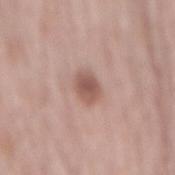notes: imaged on a skin check; not biopsied | body site: the mid back | lighting: white-light | TBP lesion metrics: a normalized border contrast of about 8; border irregularity of about 2 on a 0–10 scale and a color-variation rating of about 2.5/10 | image: ~15 mm crop, total-body skin-cancer survey | patient: male, roughly 65 years of age.